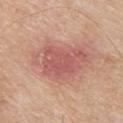| feature | finding |
|---|---|
| follow-up | imaged on a skin check; not biopsied |